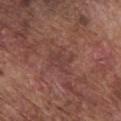follow-up: catalogued during a skin exam; not biopsied
image: ~15 mm tile from a whole-body skin photo
lesion size: about 3.5 mm
automated metrics: an area of roughly 7.5 mm², an outline eccentricity of about 0.5 (0 = round, 1 = elongated), and two-axis asymmetry of about 0.4; an average lesion color of about L≈40 a*≈22 b*≈22 (CIELAB), roughly 5 lightness units darker than nearby skin, and a normalized lesion–skin contrast near 4.5; a nevus-likeness score of about 0/100 and a detector confidence of about 95 out of 100 that the crop contains a lesion
body site: the front of the torso
patient: male, about 75 years old
tile lighting: white-light